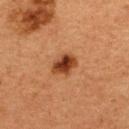The lesion was photographed on a routine skin check and not biopsied; there is no pathology result. The lesion is on the upper back. A female subject aged 38–42. This is a cross-polarized tile. Automated image analysis of the tile measured roughly 13 lightness units darker than nearby skin and a normalized lesion–skin contrast near 11.5. About 3 mm across. A lesion tile, about 15 mm wide, cut from a 3D total-body photograph.